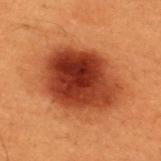Clinical impression:
Part of a total-body skin-imaging series; this lesion was reviewed on a skin check and was not flagged for biopsy.
Clinical summary:
This image is a 15 mm lesion crop taken from a total-body photograph. The tile uses cross-polarized illumination. Automated image analysis of the tile measured a footprint of about 38 mm², an eccentricity of roughly 0.6, and a symmetry-axis asymmetry near 0.15. A male patient approximately 50 years of age. The lesion is located on the back.